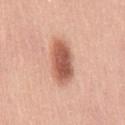workup=imaged on a skin check; not biopsied
size=~5.5 mm (longest diameter)
tile lighting=white-light
location=the lower back
subject=male, roughly 50 years of age
imaging modality=~15 mm crop, total-body skin-cancer survey
TBP lesion metrics=an average lesion color of about L≈57 a*≈25 b*≈31 (CIELAB) and a normalized border contrast of about 10; a nevus-likeness score of about 100/100 and lesion-presence confidence of about 100/100